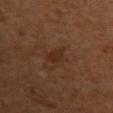Q: Is there a histopathology result?
A: no biopsy performed (imaged during a skin exam)